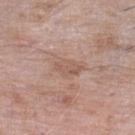Notes:
* biopsy status · total-body-photography surveillance lesion; no biopsy
* location · the left lower leg
* acquisition · total-body-photography crop, ~15 mm field of view
* automated lesion analysis · a lesion area of about 7.5 mm², an outline eccentricity of about 0.8 (0 = round, 1 = elongated), and two-axis asymmetry of about 0.4; a detector confidence of about 100 out of 100 that the crop contains a lesion
* lesion size · about 4 mm
* subject · male, in their mid-70s